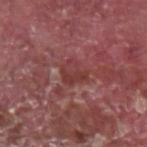The lesion was tiled from a total-body skin photograph and was not biopsied. On the left forearm. A 15 mm crop from a total-body photograph taken for skin-cancer surveillance. This is a white-light tile. Longest diameter approximately 3 mm. Automated tile analysis of the lesion measured an outline eccentricity of about 0.45 (0 = round, 1 = elongated) and a shape-asymmetry score of about 0.4 (0 = symmetric). The analysis additionally found border irregularity of about 4 on a 0–10 scale, a within-lesion color-variation index near 3.5/10, and a peripheral color-asymmetry measure near 1. And it measured a nevus-likeness score of about 0/100 and lesion-presence confidence of about 50/100. A male subject, aged 38 to 42.